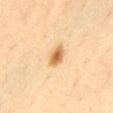From the abdomen.
This is a cross-polarized tile.
The total-body-photography lesion software estimated border irregularity of about 2 on a 0–10 scale, a color-variation rating of about 4/10, and radial color variation of about 1.5.
A male subject about 35 years old.
Measured at roughly 3 mm in maximum diameter.
Cropped from a whole-body photographic skin survey; the tile spans about 15 mm.